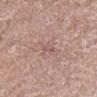No biopsy was performed on this lesion — it was imaged during a full skin examination and was not determined to be concerning. The lesion is located on the left lower leg. The subject is a male aged approximately 60. Cropped from a whole-body photographic skin survey; the tile spans about 15 mm. This is a white-light tile. The total-body-photography lesion software estimated a footprint of about 2.5 mm². It also reported a color-variation rating of about 0/10.